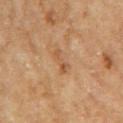Impression: Recorded during total-body skin imaging; not selected for excision or biopsy. Background: A 15 mm close-up extracted from a 3D total-body photography capture. A female patient in their 60s. This is a cross-polarized tile. The lesion is located on the right upper arm. Measured at roughly 3 mm in maximum diameter.